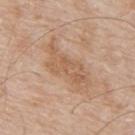Findings:
* follow-up: catalogued during a skin exam; not biopsied
* lighting: white-light illumination
* acquisition: ~15 mm tile from a whole-body skin photo
* location: the upper back
* patient: male, aged around 65
* lesion diameter: ≈6.5 mm